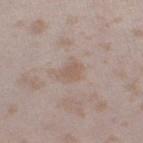follow-up = no biopsy performed (imaged during a skin exam)
subject = female, in their mid- to late 20s
body site = the left thigh
acquisition = 15 mm crop, total-body photography